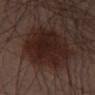– follow-up · imaged on a skin check; not biopsied
– size · ≈9.5 mm
– body site · the left thigh
– acquisition · ~15 mm crop, total-body skin-cancer survey
– subject · male, aged 38–42
– automated metrics · a lesion area of about 38 mm² and an eccentricity of roughly 0.85; a normalized lesion–skin contrast near 10.5; a color-variation rating of about 3.5/10 and a peripheral color-asymmetry measure near 1; a nevus-likeness score of about 85/100 and a detector confidence of about 100 out of 100 that the crop contains a lesion